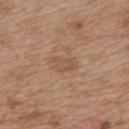{"biopsy_status": "not biopsied; imaged during a skin examination", "patient": {"sex": "female", "age_approx": 40}, "site": "back", "image": {"source": "total-body photography crop", "field_of_view_mm": 15}, "automated_metrics": {"area_mm2_approx": 4.0, "eccentricity": 0.85, "shape_asymmetry": 0.35, "cielab_L": 52, "cielab_a": 19, "cielab_b": 30, "vs_skin_darker_L": 6.0, "vs_skin_contrast_norm": 5.0, "color_variation_0_10": 2.0, "peripheral_color_asymmetry": 0.5}, "lesion_size": {"long_diameter_mm_approx": 3.0}, "lighting": "white-light"}